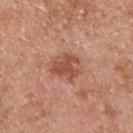notes = imaged on a skin check; not biopsied | body site = the upper back | lesion size = ~3.5 mm (longest diameter) | image source = ~15 mm tile from a whole-body skin photo | TBP lesion metrics = a footprint of about 9 mm², an eccentricity of roughly 0.5, and two-axis asymmetry of about 0.25; an average lesion color of about L≈52 a*≈25 b*≈31 (CIELAB) and a normalized border contrast of about 7; border irregularity of about 2.5 on a 0–10 scale, a color-variation rating of about 3.5/10, and peripheral color asymmetry of about 1 | lighting = white-light illumination | patient = male, aged 53–57.